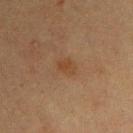| feature | finding |
|---|---|
| notes | imaged on a skin check; not biopsied |
| anatomic site | the arm |
| imaging modality | ~15 mm crop, total-body skin-cancer survey |
| tile lighting | cross-polarized |
| lesion diameter | ~2.5 mm (longest diameter) |
| patient | male, roughly 40 years of age |